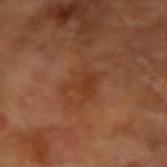Findings:
- follow-up · no biopsy performed (imaged during a skin exam)
- image · total-body-photography crop, ~15 mm field of view
- lighting · cross-polarized illumination
- lesion diameter · about 4 mm
- TBP lesion metrics · a footprint of about 6 mm², an eccentricity of roughly 0.65, and a shape-asymmetry score of about 0.4 (0 = symmetric); a lesion color around L≈36 a*≈24 b*≈32 in CIELAB, roughly 6 lightness units darker than nearby skin, and a lesion-to-skin contrast of about 5.5 (normalized; higher = more distinct); a classifier nevus-likeness of about 0/100 and a detector confidence of about 100 out of 100 that the crop contains a lesion
- subject · male, about 65 years old